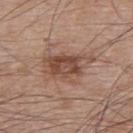notes: total-body-photography surveillance lesion; no biopsy | patient: male, roughly 65 years of age | lesion diameter: ≈6.5 mm | image: 15 mm crop, total-body photography | body site: the upper back | tile lighting: white-light illumination.